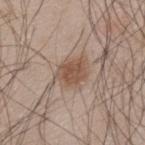Impression:
The lesion was tiled from a total-body skin photograph and was not biopsied.
Context:
A lesion tile, about 15 mm wide, cut from a 3D total-body photograph. A male subject, aged approximately 45. On the back. Approximately 4 mm at its widest. Imaged with white-light lighting.